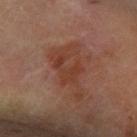notes: imaged on a skin check; not biopsied
automated lesion analysis: a lesion color around L≈35 a*≈21 b*≈26 in CIELAB and a lesion-to-skin contrast of about 6.5 (normalized; higher = more distinct); an automated nevus-likeness rating near 0 out of 100 and lesion-presence confidence of about 100/100
body site: the right forearm
acquisition: total-body-photography crop, ~15 mm field of view
subject: female, in their 60s